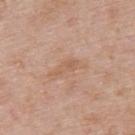• notes: catalogued during a skin exam; not biopsied
• automated lesion analysis: a footprint of about 3 mm², a shape eccentricity near 0.9, and a symmetry-axis asymmetry near 0.35; a mean CIELAB color near L≈59 a*≈20 b*≈32 and a normalized border contrast of about 5; a border-irregularity rating of about 4/10, a within-lesion color-variation index near 0.5/10, and a peripheral color-asymmetry measure near 0
• subject: male, aged 53 to 57
• illumination: white-light illumination
• body site: the upper back
• imaging modality: total-body-photography crop, ~15 mm field of view
• diameter: ≈3 mm